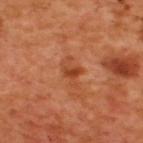Part of a total-body skin-imaging series; this lesion was reviewed on a skin check and was not flagged for biopsy.
A 15 mm close-up extracted from a 3D total-body photography capture.
Located on the upper back.
A male subject, aged 48 to 52.
The lesion's longest dimension is about 3.5 mm.